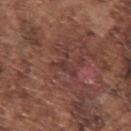No biopsy was performed on this lesion — it was imaged during a full skin examination and was not determined to be concerning. The lesion is on the left upper arm. Measured at roughly 3.5 mm in maximum diameter. Automated image analysis of the tile measured a lesion area of about 3.5 mm², a shape eccentricity near 0.8, and two-axis asymmetry of about 0.7. The analysis additionally found an average lesion color of about L≈36 a*≈22 b*≈21 (CIELAB), roughly 6 lightness units darker than nearby skin, and a normalized lesion–skin contrast near 5.5. And it measured a color-variation rating of about 0.5/10 and a peripheral color-asymmetry measure near 0. And it measured a classifier nevus-likeness of about 0/100. Cropped from a whole-body photographic skin survey; the tile spans about 15 mm. This is a white-light tile. The subject is a male about 75 years old.